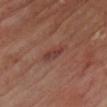biopsy_status: not biopsied; imaged during a skin examination
automated_metrics:
  cielab_L: 37
  cielab_a: 23
  cielab_b: 23
  vs_skin_darker_L: 8.0
  vs_skin_contrast_norm: 6.5
lesion_size:
  long_diameter_mm_approx: 3.0
patient:
  sex: male
  age_approx: 70
site: chest
image:
  source: total-body photography crop
  field_of_view_mm: 15